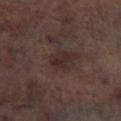Acquisition and patient details: The lesion is on the left lower leg. A roughly 15 mm field-of-view crop from a total-body skin photograph. A male subject, approximately 65 years of age. The lesion's longest dimension is about 2.5 mm. Imaged with cross-polarized lighting.